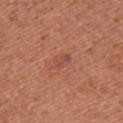notes: catalogued during a skin exam; not biopsied
subject: female, aged around 50
image-analysis metrics: a footprint of about 3.5 mm² and an eccentricity of roughly 0.8; a border-irregularity rating of about 3/10, a within-lesion color-variation index near 1.5/10, and radial color variation of about 0.5; a classifier nevus-likeness of about 5/100
lighting: white-light illumination
body site: the upper back
imaging modality: ~15 mm crop, total-body skin-cancer survey
lesion diameter: ≈2.5 mm